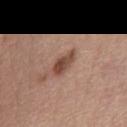workup = total-body-photography surveillance lesion; no biopsy
subject = female, aged around 55
image = ~15 mm crop, total-body skin-cancer survey
automated metrics = a lesion–skin lightness drop of about 13 and a normalized lesion–skin contrast near 9.5
illumination = white-light illumination
anatomic site = the chest